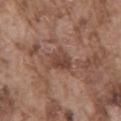biopsy status = catalogued during a skin exam; not biopsied
subject = male, approximately 75 years of age
lesion diameter = about 3 mm
acquisition = total-body-photography crop, ~15 mm field of view
illumination = white-light illumination
anatomic site = the back